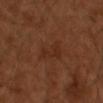The lesion was tiled from a total-body skin photograph and was not biopsied.
This image is a 15 mm lesion crop taken from a total-body photograph.
The subject is a male about 65 years old.
About 3 mm across.